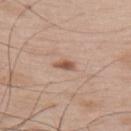follow-up: imaged on a skin check; not biopsied | patient: male, in their mid- to late 60s | site: the upper back | image source: ~15 mm tile from a whole-body skin photo.